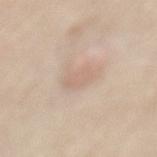Clinical impression:
Imaged during a routine full-body skin examination; the lesion was not biopsied and no histopathology is available.
Acquisition and patient details:
The recorded lesion diameter is about 3 mm. From the mid back. The tile uses white-light illumination. The patient is a female approximately 65 years of age. Cropped from a whole-body photographic skin survey; the tile spans about 15 mm.